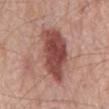Part of a total-body skin-imaging series; this lesion was reviewed on a skin check and was not flagged for biopsy.
A male subject, aged 68–72.
The lesion is on the mid back.
A region of skin cropped from a whole-body photographic capture, roughly 15 mm wide.
An algorithmic analysis of the crop reported a lesion area of about 24 mm², an outline eccentricity of about 0.9 (0 = round, 1 = elongated), and a shape-asymmetry score of about 0.15 (0 = symmetric). The analysis additionally found a mean CIELAB color near L≈48 a*≈25 b*≈24 and about 15 CIELAB-L* units darker than the surrounding skin. And it measured an automated nevus-likeness rating near 95 out of 100 and a lesion-detection confidence of about 100/100.